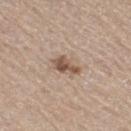Part of a total-body skin-imaging series; this lesion was reviewed on a skin check and was not flagged for biopsy. Captured under white-light illumination. A female subject aged approximately 70. The lesion-visualizer software estimated a lesion color around L≈53 a*≈16 b*≈27 in CIELAB, roughly 13 lightness units darker than nearby skin, and a normalized lesion–skin contrast near 9. The software also gave internal color variation of about 2.5 on a 0–10 scale. A 15 mm crop from a total-body photograph taken for skin-cancer surveillance. The lesion is on the right thigh. Measured at roughly 3 mm in maximum diameter.